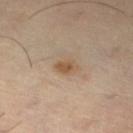Assessment: This lesion was catalogued during total-body skin photography and was not selected for biopsy. Image and clinical context: Imaged with cross-polarized lighting. A 15 mm close-up extracted from a 3D total-body photography capture. The total-body-photography lesion software estimated a border-irregularity index near 2.5/10, a color-variation rating of about 3/10, and radial color variation of about 1. Measured at roughly 3 mm in maximum diameter. On the left lower leg. A female patient, about 45 years old.